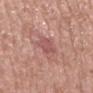The lesion was photographed on a routine skin check and not biopsied; there is no pathology result. The patient is a male approximately 65 years of age. From the mid back. A 15 mm close-up tile from a total-body photography series done for melanoma screening. Imaged with white-light lighting.